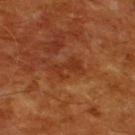imaging modality=~15 mm crop, total-body skin-cancer survey | subject=male, aged 58–62 | lighting=cross-polarized illumination | site=the back | size=~3 mm (longest diameter).